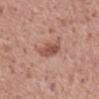Imaged during a routine full-body skin examination; the lesion was not biopsied and no histopathology is available. A roughly 15 mm field-of-view crop from a total-body skin photograph. From the mid back. A male patient, aged approximately 70.